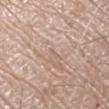Assessment: No biopsy was performed on this lesion — it was imaged during a full skin examination and was not determined to be concerning. Clinical summary: Captured under white-light illumination. A male subject, about 80 years old. A lesion tile, about 15 mm wide, cut from a 3D total-body photograph. The lesion is located on the right arm.